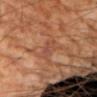notes: catalogued during a skin exam; not biopsied
patient: male, in their mid-50s
tile lighting: cross-polarized
body site: the left upper arm
automated metrics: a footprint of about 4.5 mm², an eccentricity of roughly 0.85, and two-axis asymmetry of about 0.2; a mean CIELAB color near L≈45 a*≈23 b*≈29 and a lesion-to-skin contrast of about 5 (normalized; higher = more distinct); a border-irregularity rating of about 2.5/10, a color-variation rating of about 2.5/10, and a peripheral color-asymmetry measure near 1; a classifier nevus-likeness of about 0/100 and a detector confidence of about 80 out of 100 that the crop contains a lesion
image source: total-body-photography crop, ~15 mm field of view
diameter: about 3 mm